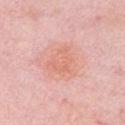The lesion's longest dimension is about 4 mm. This image is a 15 mm lesion crop taken from a total-body photograph. Captured under white-light illumination. A female patient, aged around 65. The lesion is located on the left upper arm.